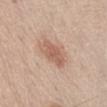Captured during whole-body skin photography for melanoma surveillance; the lesion was not biopsied.
On the abdomen.
This is a white-light tile.
A male subject, about 80 years old.
Approximately 5 mm at its widest.
Cropped from a whole-body photographic skin survey; the tile spans about 15 mm.